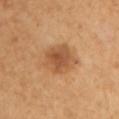{
  "biopsy_status": "not biopsied; imaged during a skin examination",
  "patient": {
    "sex": "female",
    "age_approx": 50
  },
  "site": "left upper arm",
  "image": {
    "source": "total-body photography crop",
    "field_of_view_mm": 15
  },
  "lighting": "cross-polarized",
  "automated_metrics": {
    "area_mm2_approx": 13.0,
    "shape_asymmetry": 0.15,
    "nevus_likeness_0_100": 70
  }
}